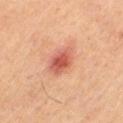Captured during whole-body skin photography for melanoma surveillance; the lesion was not biopsied. Longest diameter approximately 4 mm. The lesion is located on the left thigh. The subject is a female approximately 40 years of age. The tile uses cross-polarized illumination. A close-up tile cropped from a whole-body skin photograph, about 15 mm across.